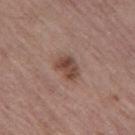Q: Is there a histopathology result?
A: no biopsy performed (imaged during a skin exam)
Q: What kind of image is this?
A: ~15 mm crop, total-body skin-cancer survey
Q: Who is the patient?
A: male, aged approximately 65
Q: What lighting was used for the tile?
A: white-light
Q: Where on the body is the lesion?
A: the left thigh